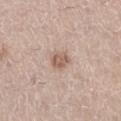| key | value |
|---|---|
| workup | catalogued during a skin exam; not biopsied |
| imaging modality | 15 mm crop, total-body photography |
| patient | female, aged approximately 40 |
| anatomic site | the leg |